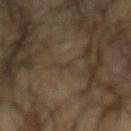Q: Was this lesion biopsied?
A: no biopsy performed (imaged during a skin exam)
Q: Where on the body is the lesion?
A: the mid back
Q: Automated lesion metrics?
A: an average lesion color of about L≈32 a*≈8 b*≈23 (CIELAB), about 3 CIELAB-L* units darker than the surrounding skin, and a normalized border contrast of about 4; a classifier nevus-likeness of about 0/100 and lesion-presence confidence of about 0/100
Q: What is the lesion's diameter?
A: ≈1.5 mm
Q: Who is the patient?
A: male, aged 63 to 67
Q: What lighting was used for the tile?
A: cross-polarized illumination
Q: What kind of image is this?
A: 15 mm crop, total-body photography